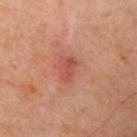<case>
<biopsy_status>not biopsied; imaged during a skin examination</biopsy_status>
<lighting>cross-polarized</lighting>
<site>left upper arm</site>
<lesion_size>
  <long_diameter_mm_approx>3.0</long_diameter_mm_approx>
</lesion_size>
<image>
  <source>total-body photography crop</source>
  <field_of_view_mm>15</field_of_view_mm>
</image>
<patient>
  <sex>male</sex>
  <age_approx>65</age_approx>
</patient>
</case>